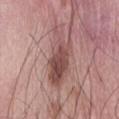No biopsy was performed on this lesion — it was imaged during a full skin examination and was not determined to be concerning. A male subject, aged 53–57. The recorded lesion diameter is about 8 mm. Located on the abdomen. A lesion tile, about 15 mm wide, cut from a 3D total-body photograph. Automated image analysis of the tile measured an area of roughly 16 mm² and a shape eccentricity near 0.95. And it measured a color-variation rating of about 5/10 and radial color variation of about 2. The tile uses white-light illumination.